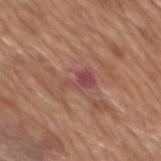notes — no biopsy performed (imaged during a skin exam)
size — about 3.5 mm
location — the back
TBP lesion metrics — a footprint of about 5 mm² and two-axis asymmetry of about 0.5; a lesion color around L≈47 a*≈26 b*≈22 in CIELAB, about 8 CIELAB-L* units darker than the surrounding skin, and a normalized border contrast of about 7.5; a color-variation rating of about 3/10 and radial color variation of about 1; a nevus-likeness score of about 0/100 and lesion-presence confidence of about 95/100
imaging modality — ~15 mm tile from a whole-body skin photo
patient — male, aged 63–67
tile lighting — white-light illumination